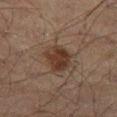workup: imaged on a skin check; not biopsied | image-analysis metrics: a shape eccentricity near 0.45 and a symmetry-axis asymmetry near 0.2; a mean CIELAB color near L≈28 a*≈15 b*≈21, about 9 CIELAB-L* units darker than the surrounding skin, and a normalized border contrast of about 9 | body site: the left thigh | image: 15 mm crop, total-body photography | subject: male, approximately 70 years of age | illumination: cross-polarized | lesion size: about 3.5 mm.